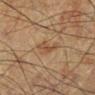biopsy_status: not biopsied; imaged during a skin examination
patient:
  sex: male
  age_approx: 60
site: left lower leg
lighting: cross-polarized
image:
  source: total-body photography crop
  field_of_view_mm: 15
automated_metrics:
  area_mm2_approx: 3.5
  eccentricity: 0.95
  shape_asymmetry: 0.5
  cielab_L: 37
  cielab_a: 15
  cielab_b: 26
  border_irregularity_0_10: 6.0
  color_variation_0_10: 0.5
  peripheral_color_asymmetry: 0.0
  nevus_likeness_0_100: 0
  lesion_detection_confidence_0_100: 80